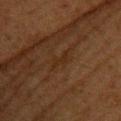biopsy_status: not biopsied; imaged during a skin examination
site: upper back
lesion_size:
  long_diameter_mm_approx: 2.5
image:
  source: total-body photography crop
  field_of_view_mm: 15
lighting: cross-polarized
patient:
  sex: female
  age_approx: 50